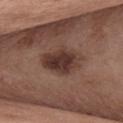Impression:
Recorded during total-body skin imaging; not selected for excision or biopsy.
Background:
Located on the front of the torso. Captured under white-light illumination. A 15 mm close-up tile from a total-body photography series done for melanoma screening. The patient is a female aged 48–52. Longest diameter approximately 5 mm.